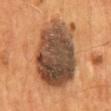Impression:
Part of a total-body skin-imaging series; this lesion was reviewed on a skin check and was not flagged for biopsy.
Clinical summary:
Approximately 9 mm at its widest. A roughly 15 mm field-of-view crop from a total-body skin photograph. The lesion is on the back. A male subject aged 53–57. The tile uses cross-polarized illumination.